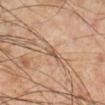Located on the right lower leg. A 15 mm close-up tile from a total-body photography series done for melanoma screening. The lesion's longest dimension is about 1.5 mm. The subject is a male about 55 years old. Captured under cross-polarized illumination.